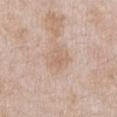Assessment:
Imaged during a routine full-body skin examination; the lesion was not biopsied and no histopathology is available.
Background:
The lesion's longest dimension is about 3 mm. A male patient, aged 48–52. The tile uses white-light illumination. A 15 mm crop from a total-body photograph taken for skin-cancer surveillance. On the chest. The total-body-photography lesion software estimated a border-irregularity index near 4.5/10, internal color variation of about 1 on a 0–10 scale, and peripheral color asymmetry of about 0.5. It also reported a classifier nevus-likeness of about 0/100 and a detector confidence of about 100 out of 100 that the crop contains a lesion.